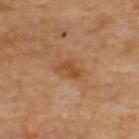biopsy status=catalogued during a skin exam; not biopsied | image-analysis metrics=a lesion area of about 5 mm², an eccentricity of roughly 0.8, and a symmetry-axis asymmetry near 0.25; a color-variation rating of about 2.5/10; a classifier nevus-likeness of about 0/100 and a lesion-detection confidence of about 100/100 | tile lighting=cross-polarized | acquisition=total-body-photography crop, ~15 mm field of view | location=the upper back.